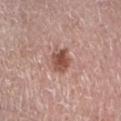Q: Is there a histopathology result?
A: no biopsy performed (imaged during a skin exam)
Q: How was this image acquired?
A: ~15 mm tile from a whole-body skin photo
Q: What lighting was used for the tile?
A: white-light
Q: Automated lesion metrics?
A: a lesion area of about 6 mm², a shape eccentricity near 0.45, and two-axis asymmetry of about 0.15; an average lesion color of about L≈51 a*≈22 b*≈27 (CIELAB), a lesion–skin lightness drop of about 13, and a normalized lesion–skin contrast near 9; an automated nevus-likeness rating near 90 out of 100 and lesion-presence confidence of about 100/100
Q: Lesion location?
A: the left lower leg
Q: Who is the patient?
A: male, aged around 75